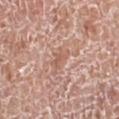Part of a total-body skin-imaging series; this lesion was reviewed on a skin check and was not flagged for biopsy. The subject is a male in their mid-70s. From the right lower leg. A 15 mm close-up tile from a total-body photography series done for melanoma screening. Imaged with white-light lighting.